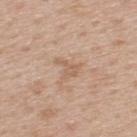Captured during whole-body skin photography for melanoma surveillance; the lesion was not biopsied. A 15 mm close-up tile from a total-body photography series done for melanoma screening. This is a white-light tile. Located on the upper back. The recorded lesion diameter is about 3 mm. The patient is a male aged approximately 60.